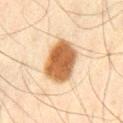Q: Is there a histopathology result?
A: total-body-photography surveillance lesion; no biopsy
Q: Illumination type?
A: cross-polarized
Q: How was this image acquired?
A: total-body-photography crop, ~15 mm field of view
Q: Where on the body is the lesion?
A: the abdomen
Q: Patient demographics?
A: male, approximately 45 years of age
Q: What did automated image analysis measure?
A: a lesion area of about 18 mm², a shape eccentricity near 0.7, and two-axis asymmetry of about 0.1; a lesion color around L≈50 a*≈18 b*≈34 in CIELAB, about 17 CIELAB-L* units darker than the surrounding skin, and a lesion-to-skin contrast of about 12 (normalized; higher = more distinct); internal color variation of about 4 on a 0–10 scale and a peripheral color-asymmetry measure near 1